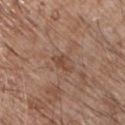Clinical impression: Captured during whole-body skin photography for melanoma surveillance; the lesion was not biopsied. Context: This is a white-light tile. The lesion is located on the chest. The subject is a male about 65 years old. Measured at roughly 3 mm in maximum diameter. A region of skin cropped from a whole-body photographic capture, roughly 15 mm wide. Automated tile analysis of the lesion measured a shape eccentricity near 0.85 and a symmetry-axis asymmetry near 0.4.